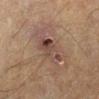  biopsy_status: not biopsied; imaged during a skin examination
  patient:
    sex: male
    age_approx: 60
  site: left lower leg
  image:
    source: total-body photography crop
    field_of_view_mm: 15